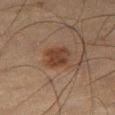Q: Was a biopsy performed?
A: catalogued during a skin exam; not biopsied
Q: What kind of image is this?
A: ~15 mm tile from a whole-body skin photo
Q: What is the lesion's diameter?
A: about 3 mm
Q: What lighting was used for the tile?
A: cross-polarized illumination
Q: Patient demographics?
A: male, approximately 65 years of age
Q: Lesion location?
A: the right thigh